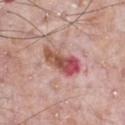The lesion was photographed on a routine skin check and not biopsied; there is no pathology result. The lesion is located on the chest. A roughly 15 mm field-of-view crop from a total-body skin photograph. A male patient aged 68–72. Imaged with white-light lighting.